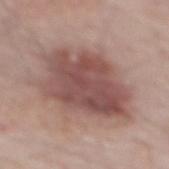| feature | finding |
|---|---|
| location | the back |
| image | ~15 mm crop, total-body skin-cancer survey |
| TBP lesion metrics | a footprint of about 41 mm², an eccentricity of roughly 0.7, and a shape-asymmetry score of about 0.2 (0 = symmetric) |
| lighting | white-light |
| lesion size | ~8.5 mm (longest diameter) |
| patient | male, in their 70s |